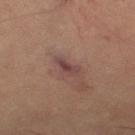Imaged during a routine full-body skin examination; the lesion was not biopsied and no histopathology is available.
The lesion is located on the right thigh.
A female subject, aged approximately 70.
This is a cross-polarized tile.
A 15 mm close-up extracted from a 3D total-body photography capture.
Longest diameter approximately 3 mm.